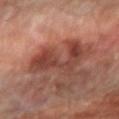No biopsy was performed on this lesion — it was imaged during a full skin examination and was not determined to be concerning. Longest diameter approximately 6 mm. A 15 mm close-up tile from a total-body photography series done for melanoma screening. The subject is a male aged 63–67. The total-body-photography lesion software estimated an average lesion color of about L≈41 a*≈25 b*≈26 (CIELAB), a lesion–skin lightness drop of about 10, and a normalized border contrast of about 8. And it measured border irregularity of about 8.5 on a 0–10 scale, a color-variation rating of about 4.5/10, and peripheral color asymmetry of about 1.5. This is a cross-polarized tile. The lesion is located on the right forearm.